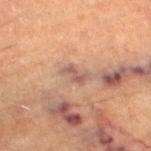Q: Is there a histopathology result?
A: catalogued during a skin exam; not biopsied
Q: Where on the body is the lesion?
A: the left thigh
Q: What is the imaging modality?
A: 15 mm crop, total-body photography
Q: Patient demographics?
A: male, aged approximately 85 or older